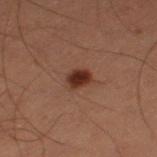Clinical impression:
Imaged during a routine full-body skin examination; the lesion was not biopsied and no histopathology is available.
Background:
The patient is a male aged approximately 60. Measured at roughly 2.5 mm in maximum diameter. Automated image analysis of the tile measured a lesion color around L≈23 a*≈18 b*≈21 in CIELAB, about 11 CIELAB-L* units darker than the surrounding skin, and a normalized lesion–skin contrast near 12. It also reported an automated nevus-likeness rating near 100 out of 100. The lesion is located on the leg. A close-up tile cropped from a whole-body skin photograph, about 15 mm across. Imaged with cross-polarized lighting.